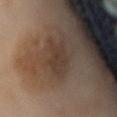This image is a 15 mm lesion crop taken from a total-body photograph. A male patient, aged 68–72. The lesion is on the mid back.